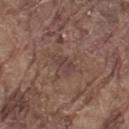Imaged during a routine full-body skin examination; the lesion was not biopsied and no histopathology is available.
On the leg.
Cropped from a total-body skin-imaging series; the visible field is about 15 mm.
A male patient, aged 78–82.
The lesion-visualizer software estimated a footprint of about 4 mm² and two-axis asymmetry of about 0.35.